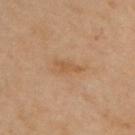biopsy_status: not biopsied; imaged during a skin examination
site: arm
image:
  source: total-body photography crop
  field_of_view_mm: 15